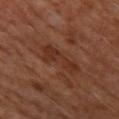Imaged during a routine full-body skin examination; the lesion was not biopsied and no histopathology is available. The lesion is located on the left thigh. A roughly 15 mm field-of-view crop from a total-body skin photograph. The tile uses cross-polarized illumination. A male subject aged around 60.